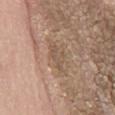{"patient": {"sex": "male", "age_approx": 75}, "lesion_size": {"long_diameter_mm_approx": 3.5}, "site": "back", "automated_metrics": {"border_irregularity_0_10": 5.0, "color_variation_0_10": 0.0, "nevus_likeness_0_100": 0, "lesion_detection_confidence_0_100": 80}, "image": {"source": "total-body photography crop", "field_of_view_mm": 15}}